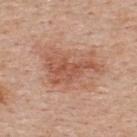Part of a total-body skin-imaging series; this lesion was reviewed on a skin check and was not flagged for biopsy. Located on the back. A male subject, aged 53–57. A lesion tile, about 15 mm wide, cut from a 3D total-body photograph. The tile uses white-light illumination. The lesion-visualizer software estimated a shape-asymmetry score of about 0.5 (0 = symmetric). It also reported border irregularity of about 6.5 on a 0–10 scale and a peripheral color-asymmetry measure near 1.5. The recorded lesion diameter is about 7 mm.